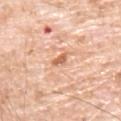No biopsy was performed on this lesion — it was imaged during a full skin examination and was not determined to be concerning.
From the back.
The subject is a male aged around 70.
Measured at roughly 2.5 mm in maximum diameter.
The lesion-visualizer software estimated an outline eccentricity of about 0.9 (0 = round, 1 = elongated). It also reported an average lesion color of about L≈64 a*≈25 b*≈36 (CIELAB), a lesion–skin lightness drop of about 12, and a lesion-to-skin contrast of about 8 (normalized; higher = more distinct). The software also gave a border-irregularity index near 5.5/10, internal color variation of about 0 on a 0–10 scale, and a peripheral color-asymmetry measure near 0.
A region of skin cropped from a whole-body photographic capture, roughly 15 mm wide.
This is a white-light tile.